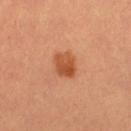Q: Is there a histopathology result?
A: catalogued during a skin exam; not biopsied
Q: Where on the body is the lesion?
A: the leg
Q: How was this image acquired?
A: ~15 mm tile from a whole-body skin photo
Q: What are the patient's age and sex?
A: female, aged around 25